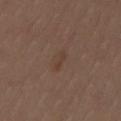Q: What is the anatomic site?
A: the left thigh
Q: What did automated image analysis measure?
A: a mean CIELAB color near L≈38 a*≈16 b*≈24 and a lesion–skin lightness drop of about 5
Q: What lighting was used for the tile?
A: white-light
Q: What kind of image is this?
A: 15 mm crop, total-body photography
Q: Lesion size?
A: ~3 mm (longest diameter)
Q: Who is the patient?
A: female, about 40 years old